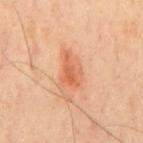workup: total-body-photography surveillance lesion; no biopsy
illumination: cross-polarized
lesion diameter: about 4 mm
location: the mid back
subject: male, aged 43–47
imaging modality: total-body-photography crop, ~15 mm field of view